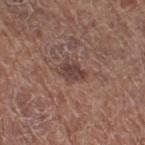This lesion was catalogued during total-body skin photography and was not selected for biopsy. The patient is a female aged approximately 50. A roughly 15 mm field-of-view crop from a total-body skin photograph. This is a white-light tile. Located on the right thigh. The lesion's longest dimension is about 2.5 mm.